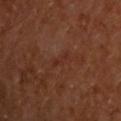notes: total-body-photography surveillance lesion; no biopsy
site: the upper back
tile lighting: cross-polarized
patient: male, in their mid- to late 60s
image source: total-body-photography crop, ~15 mm field of view
lesion size: ≈2.5 mm